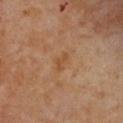| feature | finding |
|---|---|
| notes | catalogued during a skin exam; not biopsied |
| lighting | cross-polarized |
| TBP lesion metrics | an average lesion color of about L≈51 a*≈21 b*≈37 (CIELAB), roughly 6 lightness units darker than nearby skin, and a normalized lesion–skin contrast near 5.5; a lesion-detection confidence of about 100/100 |
| imaging modality | ~15 mm crop, total-body skin-cancer survey |
| lesion size | ~2.5 mm (longest diameter) |
| subject | female, in their mid-50s |
| anatomic site | the chest |